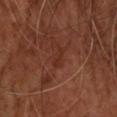No biopsy was performed on this lesion — it was imaged during a full skin examination and was not determined to be concerning.
A 15 mm close-up tile from a total-body photography series done for melanoma screening.
From the upper back.
The subject is a male aged around 55.